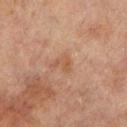Captured during whole-body skin photography for melanoma surveillance; the lesion was not biopsied.
The recorded lesion diameter is about 2.5 mm.
A male patient, about 70 years old.
Cropped from a total-body skin-imaging series; the visible field is about 15 mm.
The lesion is located on the left lower leg.
Imaged with cross-polarized lighting.
The total-body-photography lesion software estimated a lesion area of about 3 mm², an outline eccentricity of about 0.7 (0 = round, 1 = elongated), and a symmetry-axis asymmetry near 0.5. The software also gave border irregularity of about 5 on a 0–10 scale, a color-variation rating of about 1.5/10, and a peripheral color-asymmetry measure near 0.5. The analysis additionally found a lesion-detection confidence of about 100/100.